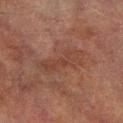Notes:
– biopsy status — catalogued during a skin exam; not biopsied
– lesion diameter — ~6 mm (longest diameter)
– tile lighting — cross-polarized illumination
– subject — male, aged around 75
– imaging modality — 15 mm crop, total-body photography
– site — the right lower leg